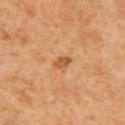Q: Was this lesion biopsied?
A: imaged on a skin check; not biopsied
Q: What are the patient's age and sex?
A: male, in their 60s
Q: How was the tile lit?
A: cross-polarized illumination
Q: What is the anatomic site?
A: the right upper arm
Q: What kind of image is this?
A: ~15 mm crop, total-body skin-cancer survey
Q: What did automated image analysis measure?
A: border irregularity of about 2 on a 0–10 scale, a within-lesion color-variation index near 1.5/10, and peripheral color asymmetry of about 0.5; a nevus-likeness score of about 35/100 and a detector confidence of about 100 out of 100 that the crop contains a lesion